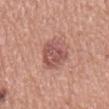Recorded during total-body skin imaging; not selected for excision or biopsy.